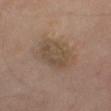* patient: male, in their mid- to late 50s
* illumination: cross-polarized illumination
* imaging modality: ~15 mm crop, total-body skin-cancer survey
* site: the right thigh
* TBP lesion metrics: an area of roughly 18 mm², an outline eccentricity of about 0.7 (0 = round, 1 = elongated), and two-axis asymmetry of about 0.2; a color-variation rating of about 3/10; a classifier nevus-likeness of about 10/100 and a lesion-detection confidence of about 100/100
* size: about 5.5 mm